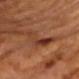Clinical impression:
The lesion was tiled from a total-body skin photograph and was not biopsied.
Context:
A male patient aged approximately 60. A close-up tile cropped from a whole-body skin photograph, about 15 mm across. The lesion's longest dimension is about 6 mm. On the upper back. Imaged with cross-polarized lighting.